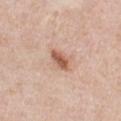follow-up: imaged on a skin check; not biopsied
lesion size: about 3 mm
subject: male, aged around 50
anatomic site: the chest
imaging modality: ~15 mm crop, total-body skin-cancer survey
tile lighting: white-light